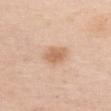Captured during whole-body skin photography for melanoma surveillance; the lesion was not biopsied.